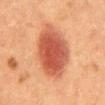No biopsy was performed on this lesion — it was imaged during a full skin examination and was not determined to be concerning. Approximately 8.5 mm at its widest. A 15 mm close-up tile from a total-body photography series done for melanoma screening. On the mid back. A female patient aged 48–52.